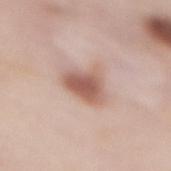Clinical impression: The lesion was photographed on a routine skin check and not biopsied; there is no pathology result. Acquisition and patient details: The recorded lesion diameter is about 3.5 mm. A roughly 15 mm field-of-view crop from a total-body skin photograph. On the back. The subject is a female about 50 years old.